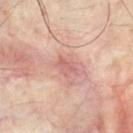  biopsy_status: not biopsied; imaged during a skin examination
  site: front of the torso
  image:
    source: total-body photography crop
    field_of_view_mm: 15
  patient:
    sex: male
    age_approx: 35